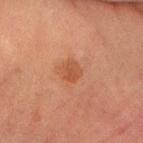A close-up tile cropped from a whole-body skin photograph, about 15 mm across.
A male subject, approximately 65 years of age.
From the right forearm.
About 2.5 mm across.